The lesion is located on the upper back · a male patient approximately 25 years of age · Automated tile analysis of the lesion measured a lesion–skin lightness drop of about 13 and a normalized border contrast of about 8.5. The analysis additionally found a classifier nevus-likeness of about 85/100 and a lesion-detection confidence of about 100/100 · a region of skin cropped from a whole-body photographic capture, roughly 15 mm wide · this is a white-light tile — 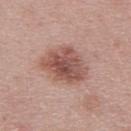Histopathological examination showed a dysplastic (Clark) nevus.